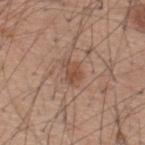This lesion was catalogued during total-body skin photography and was not selected for biopsy. The subject is a male aged 58–62. The lesion-visualizer software estimated a footprint of about 3.5 mm², an outline eccentricity of about 0.7 (0 = round, 1 = elongated), and a shape-asymmetry score of about 0.4 (0 = symmetric). A 15 mm close-up tile from a total-body photography series done for melanoma screening. The recorded lesion diameter is about 2.5 mm. From the upper back. This is a white-light tile.